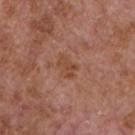About 3 mm across. Located on the chest. Captured under white-light illumination. Cropped from a total-body skin-imaging series; the visible field is about 15 mm. The lesion-visualizer software estimated a border-irregularity index near 4/10 and peripheral color asymmetry of about 0.5. The subject is a male aged around 65.